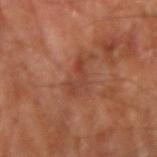The lesion was photographed on a routine skin check and not biopsied; there is no pathology result.
A male subject, roughly 70 years of age.
Approximately 4 mm at its widest.
Captured under cross-polarized illumination.
A region of skin cropped from a whole-body photographic capture, roughly 15 mm wide.
The lesion is on the arm.